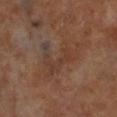No biopsy was performed on this lesion — it was imaged during a full skin examination and was not determined to be concerning.
On the right lower leg.
Measured at roughly 6.5 mm in maximum diameter.
The subject is a male in their 70s.
Cropped from a total-body skin-imaging series; the visible field is about 15 mm.
The lesion-visualizer software estimated a lesion area of about 12 mm², an outline eccentricity of about 0.8 (0 = round, 1 = elongated), and a symmetry-axis asymmetry near 0.65. It also reported a lesion color around L≈36 a*≈17 b*≈24 in CIELAB, a lesion–skin lightness drop of about 5, and a lesion-to-skin contrast of about 5 (normalized; higher = more distinct). It also reported a border-irregularity rating of about 9.5/10, a color-variation rating of about 5/10, and radial color variation of about 2.